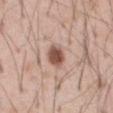follow-up — imaged on a skin check; not biopsied
automated lesion analysis — border irregularity of about 1.5 on a 0–10 scale and a within-lesion color-variation index near 3/10; a classifier nevus-likeness of about 100/100 and a detector confidence of about 100 out of 100 that the crop contains a lesion
body site — the abdomen
image source — total-body-photography crop, ~15 mm field of view
subject — male, aged approximately 55